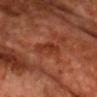Q: What is the anatomic site?
A: the front of the torso
Q: How large is the lesion?
A: ~3.5 mm (longest diameter)
Q: What did automated image analysis measure?
A: an average lesion color of about L≈35 a*≈28 b*≈33 (CIELAB), a lesion–skin lightness drop of about 7, and a lesion-to-skin contrast of about 7 (normalized; higher = more distinct)
Q: What kind of image is this?
A: ~15 mm tile from a whole-body skin photo
Q: What lighting was used for the tile?
A: cross-polarized illumination
Q: Who is the patient?
A: male, about 70 years old